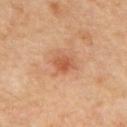Findings:
– follow-up: total-body-photography surveillance lesion; no biopsy
– image: ~15 mm tile from a whole-body skin photo
– diameter: about 2 mm
– subject: male, aged 53 to 57
– site: the upper back
– illumination: cross-polarized illumination